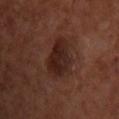Part of a total-body skin-imaging series; this lesion was reviewed on a skin check and was not flagged for biopsy. From the chest. About 5.5 mm across. Imaged with cross-polarized lighting. A lesion tile, about 15 mm wide, cut from a 3D total-body photograph. A male patient, approximately 70 years of age.